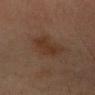Part of a total-body skin-imaging series; this lesion was reviewed on a skin check and was not flagged for biopsy.
The subject is a male aged around 35.
The lesion is on the head or neck.
Cropped from a whole-body photographic skin survey; the tile spans about 15 mm.
About 4 mm across.
This is a cross-polarized tile.
An algorithmic analysis of the crop reported a lesion area of about 7.5 mm², an eccentricity of roughly 0.75, and two-axis asymmetry of about 0.3. It also reported a mean CIELAB color near L≈29 a*≈16 b*≈24, roughly 6 lightness units darker than nearby skin, and a normalized lesion–skin contrast near 6.5. The analysis additionally found a nevus-likeness score of about 15/100 and lesion-presence confidence of about 100/100.